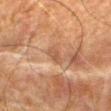<lesion>
  <lesion_size>
    <long_diameter_mm_approx>2.5</long_diameter_mm_approx>
  </lesion_size>
  <site>abdomen</site>
  <patient>
    <sex>male</sex>
    <age_approx>80</age_approx>
  </patient>
  <image>
    <source>total-body photography crop</source>
    <field_of_view_mm>15</field_of_view_mm>
  </image>
</lesion>